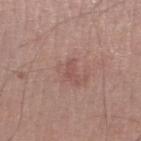<case>
<biopsy_status>not biopsied; imaged during a skin examination</biopsy_status>
<site>left lower leg</site>
<automated_metrics>
  <cielab_L>51</cielab_L>
  <cielab_a>22</cielab_a>
  <cielab_b>23</cielab_b>
  <border_irregularity_0_10>3.5</border_irregularity_0_10>
  <color_variation_0_10>0.0</color_variation_0_10>
</automated_metrics>
<patient>
  <sex>male</sex>
  <age_approx>45</age_approx>
</patient>
<image>
  <source>total-body photography crop</source>
  <field_of_view_mm>15</field_of_view_mm>
</image>
<lesion_size>
  <long_diameter_mm_approx>2.0</long_diameter_mm_approx>
</lesion_size>
<lighting>white-light</lighting>
</case>